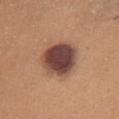Image and clinical context: A 15 mm close-up extracted from a 3D total-body photography capture. The subject is a female aged 33 to 37. The lesion is on the chest.